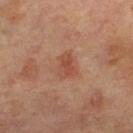Q: Is there a histopathology result?
A: catalogued during a skin exam; not biopsied
Q: Patient demographics?
A: female, aged approximately 65
Q: What is the anatomic site?
A: the left leg
Q: What is the imaging modality?
A: ~15 mm crop, total-body skin-cancer survey